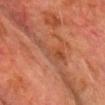Longest diameter approximately 4.5 mm.
The lesion is located on the chest.
Automated image analysis of the tile measured an area of roughly 8 mm². It also reported a normalized lesion–skin contrast near 4.5.
The subject is a male in their mid- to late 70s.
A region of skin cropped from a whole-body photographic capture, roughly 15 mm wide.